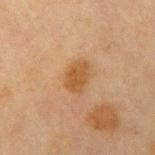Clinical impression:
Imaged during a routine full-body skin examination; the lesion was not biopsied and no histopathology is available.
Background:
The lesion is on the left upper arm. This is a cross-polarized tile. A region of skin cropped from a whole-body photographic capture, roughly 15 mm wide. The patient is a male aged 63 to 67. Longest diameter approximately 3.5 mm.